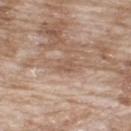Image and clinical context:
From the upper back. The subject is a male in their mid-60s. A close-up tile cropped from a whole-body skin photograph, about 15 mm across.